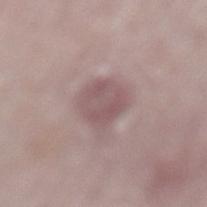Findings:
– follow-up · total-body-photography surveillance lesion; no biopsy
– body site · the left lower leg
– illumination · white-light illumination
– diameter · ≈3.5 mm
– subject · female, aged around 60
– imaging modality · ~15 mm crop, total-body skin-cancer survey
– automated lesion analysis · a mean CIELAB color near L≈53 a*≈18 b*≈16, about 9 CIELAB-L* units darker than the surrounding skin, and a normalized border contrast of about 6.5; a border-irregularity rating of about 1.5/10, internal color variation of about 2.5 on a 0–10 scale, and radial color variation of about 1